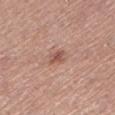• notes: total-body-photography surveillance lesion; no biopsy
• acquisition: total-body-photography crop, ~15 mm field of view
• patient: female, roughly 50 years of age
• size: ~2.5 mm (longest diameter)
• site: the left lower leg
• TBP lesion metrics: a border-irregularity rating of about 3/10, internal color variation of about 2 on a 0–10 scale, and a peripheral color-asymmetry measure near 0.5